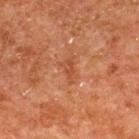Clinical impression: Imaged during a routine full-body skin examination; the lesion was not biopsied and no histopathology is available. Context: A male subject approximately 80 years of age. A lesion tile, about 15 mm wide, cut from a 3D total-body photograph. From the back. Automated tile analysis of the lesion measured a lesion area of about 3.5 mm² and two-axis asymmetry of about 0.5. The analysis additionally found a border-irregularity rating of about 5.5/10 and a within-lesion color-variation index near 1/10. The lesion's longest dimension is about 3 mm. The tile uses cross-polarized illumination.